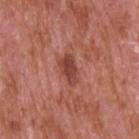Q: Was this lesion biopsied?
A: catalogued during a skin exam; not biopsied
Q: Who is the patient?
A: male, approximately 65 years of age
Q: Automated lesion metrics?
A: an average lesion color of about L≈44 a*≈27 b*≈28 (CIELAB), a lesion–skin lightness drop of about 11, and a normalized lesion–skin contrast near 8
Q: What kind of image is this?
A: ~15 mm tile from a whole-body skin photo
Q: What is the lesion's diameter?
A: ≈3.5 mm
Q: Illumination type?
A: white-light illumination
Q: Where on the body is the lesion?
A: the upper back